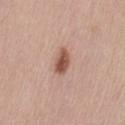<tbp_lesion>
  <biopsy_status>not biopsied; imaged during a skin examination</biopsy_status>
  <site>left thigh</site>
  <patient>
    <sex>female</sex>
    <age_approx>65</age_approx>
  </patient>
  <automated_metrics>
    <cielab_L>53</cielab_L>
    <cielab_a>22</cielab_a>
    <cielab_b>27</cielab_b>
    <vs_skin_darker_L>14.0</vs_skin_darker_L>
    <vs_skin_contrast_norm>9.5</vs_skin_contrast_norm>
    <border_irregularity_0_10>1.5</border_irregularity_0_10>
    <color_variation_0_10>3.0</color_variation_0_10>
    <peripheral_color_asymmetry>1.0</peripheral_color_asymmetry>
  </automated_metrics>
  <image>
    <source>total-body photography crop</source>
    <field_of_view_mm>15</field_of_view_mm>
  </image>
  <lighting>white-light</lighting>
</tbp_lesion>